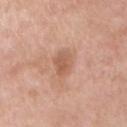The lesion was tiled from a total-body skin photograph and was not biopsied.
The tile uses white-light illumination.
A lesion tile, about 15 mm wide, cut from a 3D total-body photograph.
The lesion's longest dimension is about 2.5 mm.
On the front of the torso.
Automated tile analysis of the lesion measured a lesion area of about 5 mm², a shape eccentricity near 0.55, and a symmetry-axis asymmetry near 0.2. And it measured a border-irregularity rating of about 2/10, internal color variation of about 2 on a 0–10 scale, and radial color variation of about 1. It also reported an automated nevus-likeness rating near 0 out of 100.
A male patient, aged 53–57.